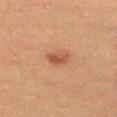Impression: Part of a total-body skin-imaging series; this lesion was reviewed on a skin check and was not flagged for biopsy. Context: Cropped from a whole-body photographic skin survey; the tile spans about 15 mm. On the left thigh. A female patient approximately 55 years of age. The recorded lesion diameter is about 3 mm. The total-body-photography lesion software estimated an area of roughly 4 mm², a shape eccentricity near 0.85, and a shape-asymmetry score of about 0.2 (0 = symmetric). The tile uses cross-polarized illumination.